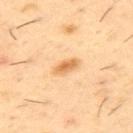No biopsy was performed on this lesion — it was imaged during a full skin examination and was not determined to be concerning. A male patient, roughly 55 years of age. A 15 mm crop from a total-body photograph taken for skin-cancer surveillance. From the upper back. Approximately 3 mm at its widest. The tile uses cross-polarized illumination.